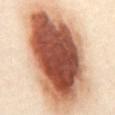| field | value |
|---|---|
| biopsy status | catalogued during a skin exam; not biopsied |
| image source | ~15 mm tile from a whole-body skin photo |
| subject | female, aged around 40 |
| image-analysis metrics | an area of roughly 100 mm², an outline eccentricity of about 0.85 (0 = round, 1 = elongated), and a symmetry-axis asymmetry near 0.15; an automated nevus-likeness rating near 100 out of 100 and a detector confidence of about 100 out of 100 that the crop contains a lesion |
| size | ~16 mm (longest diameter) |
| body site | the abdomen |
| lighting | cross-polarized |